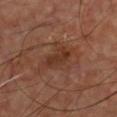follow-up: imaged on a skin check; not biopsied
image source: total-body-photography crop, ~15 mm field of view
patient: male, about 65 years old
body site: the chest